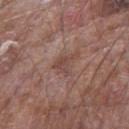Clinical impression: Captured during whole-body skin photography for melanoma surveillance; the lesion was not biopsied. Clinical summary: The tile uses white-light illumination. About 3 mm across. A lesion tile, about 15 mm wide, cut from a 3D total-body photograph. Automated image analysis of the tile measured an outline eccentricity of about 0.7 (0 = round, 1 = elongated) and a shape-asymmetry score of about 0.6 (0 = symmetric). The analysis additionally found a border-irregularity index near 7/10 and radial color variation of about 1. It also reported a classifier nevus-likeness of about 0/100 and lesion-presence confidence of about 100/100. Located on the arm. A male subject, in their mid-60s.